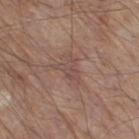<lesion>
<biopsy_status>not biopsied; imaged during a skin examination</biopsy_status>
<image>
  <source>total-body photography crop</source>
  <field_of_view_mm>15</field_of_view_mm>
</image>
<site>right thigh</site>
<patient>
  <sex>male</sex>
  <age_approx>80</age_approx>
</patient>
</lesion>